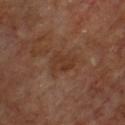Findings:
- biopsy status — imaged on a skin check; not biopsied
- lesion diameter — about 3.5 mm
- location — the front of the torso
- image source — total-body-photography crop, ~15 mm field of view
- subject — male, aged approximately 65